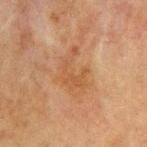follow-up: total-body-photography surveillance lesion; no biopsy
image source: ~15 mm tile from a whole-body skin photo
patient: male, roughly 65 years of age
anatomic site: the chest